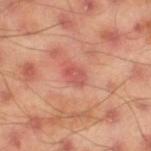• workup: no biopsy performed (imaged during a skin exam)
• automated lesion analysis: a border-irregularity index near 2/10, a color-variation rating of about 3.5/10, and radial color variation of about 1.5
• image source: total-body-photography crop, ~15 mm field of view
• subject: male, aged approximately 45
• size: about 2.5 mm
• site: the right thigh